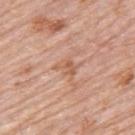workup=total-body-photography surveillance lesion; no biopsy